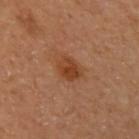biopsy status: imaged on a skin check; not biopsied
patient: female, aged 58 to 62
body site: the right arm
tile lighting: cross-polarized illumination
lesion size: ~3 mm (longest diameter)
acquisition: 15 mm crop, total-body photography